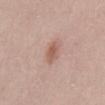| field | value |
|---|---|
| notes | imaged on a skin check; not biopsied |
| imaging modality | 15 mm crop, total-body photography |
| tile lighting | white-light illumination |
| anatomic site | the abdomen |
| diameter | ~3 mm (longest diameter) |
| patient | female, about 30 years old |